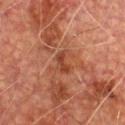The lesion was tiled from a total-body skin photograph and was not biopsied. A 15 mm close-up tile from a total-body photography series done for melanoma screening. A male patient roughly 75 years of age. This is a cross-polarized tile. The lesion is on the chest. About 3 mm across.